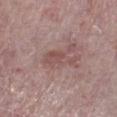<case>
  <biopsy_status>not biopsied; imaged during a skin examination</biopsy_status>
  <site>leg</site>
  <image>
    <source>total-body photography crop</source>
    <field_of_view_mm>15</field_of_view_mm>
  </image>
  <patient>
    <sex>male</sex>
    <age_approx>75</age_approx>
  </patient>
</case>